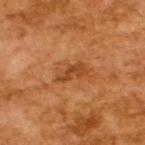Clinical impression: No biopsy was performed on this lesion — it was imaged during a full skin examination and was not determined to be concerning. Background: The subject is a male in their mid- to late 60s. Automated tile analysis of the lesion measured an area of roughly 5 mm² and a shape-asymmetry score of about 0.35 (0 = symmetric). It also reported an average lesion color of about L≈45 a*≈27 b*≈40 (CIELAB) and a lesion–skin lightness drop of about 9. The software also gave an automated nevus-likeness rating near 20 out of 100 and a lesion-detection confidence of about 100/100. About 3.5 mm across. Cropped from a total-body skin-imaging series; the visible field is about 15 mm. Captured under cross-polarized illumination.